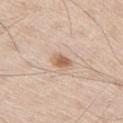Q: Was a biopsy performed?
A: total-body-photography surveillance lesion; no biopsy
Q: Illumination type?
A: white-light
Q: What kind of image is this?
A: total-body-photography crop, ~15 mm field of view
Q: Lesion size?
A: ~2.5 mm (longest diameter)
Q: Automated lesion metrics?
A: an area of roughly 4 mm², an eccentricity of roughly 0.7, and a symmetry-axis asymmetry near 0.15; border irregularity of about 1.5 on a 0–10 scale, internal color variation of about 3.5 on a 0–10 scale, and peripheral color asymmetry of about 1; a classifier nevus-likeness of about 90/100 and a lesion-detection confidence of about 100/100
Q: What are the patient's age and sex?
A: male, aged around 60
Q: What is the anatomic site?
A: the left thigh